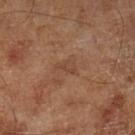- tile lighting: cross-polarized illumination
- image: ~15 mm crop, total-body skin-cancer survey
- diameter: about 2.5 mm
- patient: male, aged 63–67